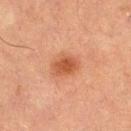Context: On the left thigh. A male patient approximately 65 years of age. A 15 mm crop from a total-body photograph taken for skin-cancer surveillance. Longest diameter approximately 3.5 mm.